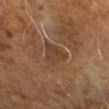Q: Was a biopsy performed?
A: imaged on a skin check; not biopsied
Q: What kind of image is this?
A: total-body-photography crop, ~15 mm field of view
Q: Automated lesion metrics?
A: a lesion color around L≈32 a*≈14 b*≈24 in CIELAB and a lesion–skin lightness drop of about 6; a border-irregularity rating of about 4.5/10, internal color variation of about 2.5 on a 0–10 scale, and radial color variation of about 1; a detector confidence of about 70 out of 100 that the crop contains a lesion
Q: How was the tile lit?
A: cross-polarized illumination
Q: Where on the body is the lesion?
A: the right forearm
Q: Who is the patient?
A: male, aged 68–72